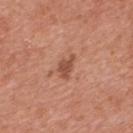Notes:
– notes — no biopsy performed (imaged during a skin exam)
– acquisition — ~15 mm tile from a whole-body skin photo
– location — the back
– patient — male, roughly 70 years of age